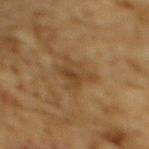biopsy status: imaged on a skin check; not biopsied | patient: male, aged 83 or older | body site: the upper back | image: ~15 mm crop, total-body skin-cancer survey.